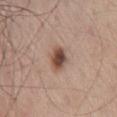This lesion was catalogued during total-body skin photography and was not selected for biopsy.
A 15 mm close-up extracted from a 3D total-body photography capture.
On the mid back.
The patient is a male about 70 years old.